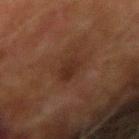follow-up=imaged on a skin check; not biopsied
image source=~15 mm tile from a whole-body skin photo
patient=male, aged approximately 75
site=the right upper arm